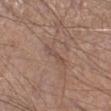  lighting: white-light
  patient:
    sex: male
    age_approx: 55
  image:
    source: total-body photography crop
    field_of_view_mm: 15
  site: left lower leg